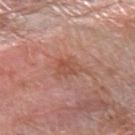notes: imaged on a skin check; not biopsied
image: 15 mm crop, total-body photography
site: the left forearm
lesion size: ≈2.5 mm
automated metrics: a shape eccentricity near 0.6 and a shape-asymmetry score of about 0.3 (0 = symmetric); a lesion-to-skin contrast of about 6 (normalized; higher = more distinct)
patient: female, aged 38 to 42
tile lighting: white-light